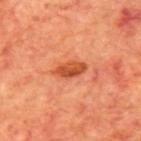– follow-up · catalogued during a skin exam; not biopsied
– anatomic site · the mid back
– imaging modality · ~15 mm crop, total-body skin-cancer survey
– subject · male, aged 68–72